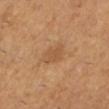Q: Was this lesion biopsied?
A: imaged on a skin check; not biopsied
Q: Automated lesion metrics?
A: an average lesion color of about L≈53 a*≈21 b*≈36 (CIELAB), a lesion–skin lightness drop of about 7, and a normalized border contrast of about 5; a classifier nevus-likeness of about 0/100 and a detector confidence of about 100 out of 100 that the crop contains a lesion
Q: How was this image acquired?
A: 15 mm crop, total-body photography
Q: What lighting was used for the tile?
A: cross-polarized
Q: How large is the lesion?
A: ~3 mm (longest diameter)
Q: Who is the patient?
A: female, in their mid- to late 50s
Q: What is the anatomic site?
A: the leg